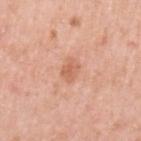<lesion>
  <biopsy_status>not biopsied; imaged during a skin examination</biopsy_status>
  <patient>
    <sex>female</sex>
    <age_approx>40</age_approx>
  </patient>
  <image>
    <source>total-body photography crop</source>
    <field_of_view_mm>15</field_of_view_mm>
  </image>
  <lighting>white-light</lighting>
  <site>left upper arm</site>
</lesion>